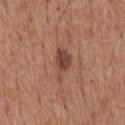A male patient aged approximately 60. The lesion is on the mid back. A 15 mm close-up extracted from a 3D total-body photography capture. Longest diameter approximately 4.5 mm. This is a white-light tile. The lesion-visualizer software estimated a border-irregularity index near 4/10 and a color-variation rating of about 3.5/10. The software also gave a detector confidence of about 100 out of 100 that the crop contains a lesion.